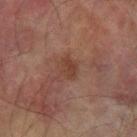<tbp_lesion>
<biopsy_status>not biopsied; imaged during a skin examination</biopsy_status>
<image>
  <source>total-body photography crop</source>
  <field_of_view_mm>15</field_of_view_mm>
</image>
<patient>
  <sex>male</sex>
  <age_approx>75</age_approx>
</patient>
<automated_metrics>
  <area_mm2_approx>4.0</area_mm2_approx>
  <eccentricity>0.75</eccentricity>
  <shape_asymmetry>0.25</shape_asymmetry>
  <vs_skin_darker_L>6.0</vs_skin_darker_L>
  <vs_skin_contrast_norm>6.0</vs_skin_contrast_norm>
  <color_variation_0_10>2.0</color_variation_0_10>
  <peripheral_color_asymmetry>1.0</peripheral_color_asymmetry>
  <nevus_likeness_0_100>0</nevus_likeness_0_100>
  <lesion_detection_confidence_0_100>100</lesion_detection_confidence_0_100>
</automated_metrics>
<site>left forearm</site>
</tbp_lesion>